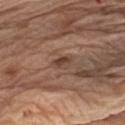Captured during whole-body skin photography for melanoma surveillance; the lesion was not biopsied.
The patient is a male aged 68–72.
The lesion is on the arm.
A close-up tile cropped from a whole-body skin photograph, about 15 mm across.
About 2.5 mm across.